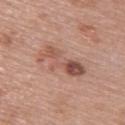Case summary:
– follow-up · catalogued during a skin exam; not biopsied